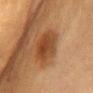This lesion was catalogued during total-body skin photography and was not selected for biopsy.
The recorded lesion diameter is about 5 mm.
A female subject approximately 55 years of age.
The tile uses cross-polarized illumination.
From the chest.
A region of skin cropped from a whole-body photographic capture, roughly 15 mm wide.
Automated tile analysis of the lesion measured a lesion area of about 13 mm². The software also gave a border-irregularity index near 2/10, internal color variation of about 4.5 on a 0–10 scale, and a peripheral color-asymmetry measure near 1.5.